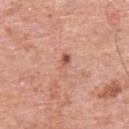| field | value |
|---|---|
| notes | imaged on a skin check; not biopsied |
| automated metrics | a shape eccentricity near 0.9 and a shape-asymmetry score of about 0.3 (0 = symmetric); a color-variation rating of about 4/10 and a peripheral color-asymmetry measure near 1; a nevus-likeness score of about 0/100 |
| subject | male, aged approximately 80 |
| lesion size | ~3.5 mm (longest diameter) |
| anatomic site | the upper back |
| image source | ~15 mm tile from a whole-body skin photo |